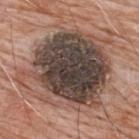Impression:
The lesion was photographed on a routine skin check and not biopsied; there is no pathology result.
Image and clinical context:
The lesion-visualizer software estimated a footprint of about 65 mm², a shape eccentricity near 0.5, and a shape-asymmetry score of about 0.25 (0 = symmetric). The analysis additionally found an average lesion color of about L≈43 a*≈14 b*≈21 (CIELAB), a lesion–skin lightness drop of about 17, and a lesion-to-skin contrast of about 13 (normalized; higher = more distinct). The software also gave a within-lesion color-variation index near 9/10 and peripheral color asymmetry of about 2.5. The recorded lesion diameter is about 10 mm. Cropped from a total-body skin-imaging series; the visible field is about 15 mm. The subject is a male aged 78 to 82. Located on the upper back.